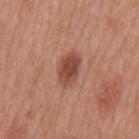{
  "biopsy_status": "not biopsied; imaged during a skin examination",
  "automated_metrics": {
    "cielab_L": 47,
    "cielab_a": 25,
    "cielab_b": 28,
    "vs_skin_contrast_norm": 8.5
  },
  "site": "mid back",
  "lighting": "white-light",
  "lesion_size": {
    "long_diameter_mm_approx": 4.0
  },
  "patient": {
    "sex": "male",
    "age_approx": 70
  },
  "image": {
    "source": "total-body photography crop",
    "field_of_view_mm": 15
  }
}